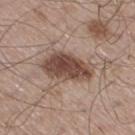Assessment:
Imaged during a routine full-body skin examination; the lesion was not biopsied and no histopathology is available.